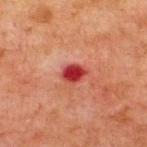Findings:
- follow-up: imaged on a skin check; not biopsied
- body site: the back
- imaging modality: ~15 mm tile from a whole-body skin photo
- subject: male, in their 60s